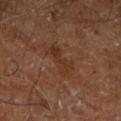Impression:
The lesion was tiled from a total-body skin photograph and was not biopsied.
Background:
Imaged with cross-polarized lighting. The lesion is located on the right leg. A male patient, approximately 60 years of age. A region of skin cropped from a whole-body photographic capture, roughly 15 mm wide. The lesion's longest dimension is about 4 mm. Automated tile analysis of the lesion measured a mean CIELAB color near L≈31 a*≈20 b*≈28, roughly 5 lightness units darker than nearby skin, and a lesion-to-skin contrast of about 6 (normalized; higher = more distinct). The software also gave a border-irregularity rating of about 5/10, internal color variation of about 2.5 on a 0–10 scale, and a peripheral color-asymmetry measure near 1.